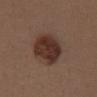notes = total-body-photography surveillance lesion; no biopsy
imaging modality = total-body-photography crop, ~15 mm field of view
diameter = about 6 mm
body site = the chest
subject = male, aged around 40